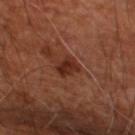Assessment: No biopsy was performed on this lesion — it was imaged during a full skin examination and was not determined to be concerning.